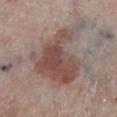Recorded during total-body skin imaging; not selected for excision or biopsy. An algorithmic analysis of the crop reported a lesion area of about 32 mm² and an outline eccentricity of about 0.5 (0 = round, 1 = elongated). The software also gave about 11 CIELAB-L* units darker than the surrounding skin and a normalized lesion–skin contrast near 8. It also reported a border-irregularity rating of about 7/10. The analysis additionally found a classifier nevus-likeness of about 75/100 and a lesion-detection confidence of about 100/100. The patient is a male aged 78–82. The lesion's longest dimension is about 9 mm. On the left lower leg. A 15 mm crop from a total-body photograph taken for skin-cancer surveillance. Captured under white-light illumination.